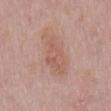{
  "biopsy_status": "not biopsied; imaged during a skin examination",
  "patient": {
    "sex": "male",
    "age_approx": 75
  },
  "lighting": "white-light",
  "image": {
    "source": "total-body photography crop",
    "field_of_view_mm": 15
  },
  "site": "mid back"
}